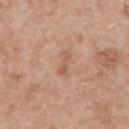Captured during whole-body skin photography for melanoma surveillance; the lesion was not biopsied. The lesion is on the chest. Imaged with white-light lighting. The patient is a male aged 48 to 52. An algorithmic analysis of the crop reported an area of roughly 2 mm², an outline eccentricity of about 0.95 (0 = round, 1 = elongated), and two-axis asymmetry of about 0.45. The recorded lesion diameter is about 3 mm. A close-up tile cropped from a whole-body skin photograph, about 15 mm across.